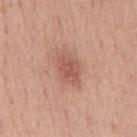Part of a total-body skin-imaging series; this lesion was reviewed on a skin check and was not flagged for biopsy.
On the back.
A male patient aged approximately 50.
This image is a 15 mm lesion crop taken from a total-body photograph.